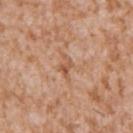workup: total-body-photography surveillance lesion; no biopsy | tile lighting: white-light | location: the arm | TBP lesion metrics: an outline eccentricity of about 0.8 (0 = round, 1 = elongated) and a symmetry-axis asymmetry near 0.45; a mean CIELAB color near L≈55 a*≈22 b*≈34 and a normalized border contrast of about 6.5; a nevus-likeness score of about 0/100 and lesion-presence confidence of about 100/100 | acquisition: total-body-photography crop, ~15 mm field of view | subject: male, approximately 45 years of age | diameter: ≈2.5 mm.